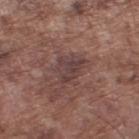No biopsy was performed on this lesion — it was imaged during a full skin examination and was not determined to be concerning. From the leg. A male subject, in their mid-70s. A 15 mm close-up tile from a total-body photography series done for melanoma screening. The lesion's longest dimension is about 4 mm. The total-body-photography lesion software estimated a lesion area of about 8 mm², an eccentricity of roughly 0.65, and a symmetry-axis asymmetry near 0.35. The software also gave a border-irregularity rating of about 4/10, internal color variation of about 3 on a 0–10 scale, and radial color variation of about 1.